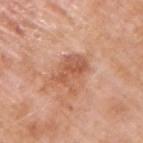A 15 mm close-up extracted from a 3D total-body photography capture. From the right upper arm. The recorded lesion diameter is about 4 mm. The subject is a male aged approximately 60. Automated tile analysis of the lesion measured a footprint of about 8.5 mm², an eccentricity of roughly 0.7, and a symmetry-axis asymmetry near 0.35. And it measured a lesion color around L≈57 a*≈25 b*≈33 in CIELAB and roughly 10 lightness units darker than nearby skin. The software also gave border irregularity of about 5 on a 0–10 scale, a color-variation rating of about 3.5/10, and a peripheral color-asymmetry measure near 1.5. It also reported an automated nevus-likeness rating near 10 out of 100.